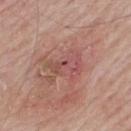Background:
Cropped from a total-body skin-imaging series; the visible field is about 15 mm. This is a white-light tile. The patient is a male roughly 65 years of age. Located on the mid back.